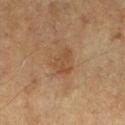The lesion was photographed on a routine skin check and not biopsied; there is no pathology result. A male patient, aged 83 or over. On the left lower leg. The lesion's longest dimension is about 3.5 mm. A lesion tile, about 15 mm wide, cut from a 3D total-body photograph. Captured under cross-polarized illumination.